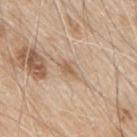Imaged during a routine full-body skin examination; the lesion was not biopsied and no histopathology is available. The lesion is on the upper back. A region of skin cropped from a whole-body photographic capture, roughly 15 mm wide. The tile uses white-light illumination. About 3 mm across. The total-body-photography lesion software estimated a border-irregularity rating of about 3.5/10 and radial color variation of about 0.5. And it measured a classifier nevus-likeness of about 0/100 and a detector confidence of about 100 out of 100 that the crop contains a lesion. A male patient aged approximately 80.